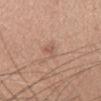Impression:
Imaged during a routine full-body skin examination; the lesion was not biopsied and no histopathology is available.
Clinical summary:
A region of skin cropped from a whole-body photographic capture, roughly 15 mm wide. The lesion is on the head or neck. A female patient, aged around 35.